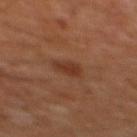<lesion>
  <biopsy_status>not biopsied; imaged during a skin examination</biopsy_status>
  <patient>
    <sex>male</sex>
    <age_approx>60</age_approx>
  </patient>
  <site>chest</site>
  <lesion_size>
    <long_diameter_mm_approx>2.5</long_diameter_mm_approx>
  </lesion_size>
  <image>
    <source>total-body photography crop</source>
    <field_of_view_mm>15</field_of_view_mm>
  </image>
</lesion>